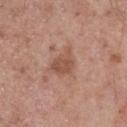Recorded during total-body skin imaging; not selected for excision or biopsy.
The lesion is on the mid back.
The total-body-photography lesion software estimated an area of roughly 5.5 mm², an outline eccentricity of about 0.7 (0 = round, 1 = elongated), and two-axis asymmetry of about 0.35. The analysis additionally found an average lesion color of about L≈51 a*≈23 b*≈28 (CIELAB), about 9 CIELAB-L* units darker than the surrounding skin, and a normalized lesion–skin contrast near 6.5. The analysis additionally found a border-irregularity index near 3.5/10, a within-lesion color-variation index near 2/10, and radial color variation of about 0.5.
A male patient aged around 55.
A 15 mm crop from a total-body photograph taken for skin-cancer surveillance.
This is a white-light tile.
About 3.5 mm across.